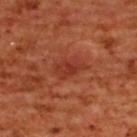Case summary:
* follow-up · catalogued during a skin exam; not biopsied
* tile lighting · cross-polarized illumination
* TBP lesion metrics · an automated nevus-likeness rating near 0 out of 100 and lesion-presence confidence of about 100/100
* lesion size · ≈3.5 mm
* subject · female, aged around 55
* site · the upper back
* image source · ~15 mm tile from a whole-body skin photo